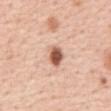<record>
<image>
  <source>total-body photography crop</source>
  <field_of_view_mm>15</field_of_view_mm>
</image>
<patient>
  <sex>female</sex>
  <age_approx>45</age_approx>
</patient>
<site>abdomen</site>
</record>